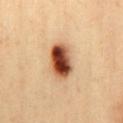Impression:
Captured during whole-body skin photography for melanoma surveillance; the lesion was not biopsied.
Acquisition and patient details:
Cropped from a whole-body photographic skin survey; the tile spans about 15 mm. Captured under cross-polarized illumination. Automated image analysis of the tile measured a lesion–skin lightness drop of about 24 and a normalized lesion–skin contrast near 14.5. From the back. About 4.5 mm across. A male patient aged around 40.